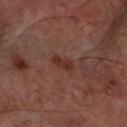The lesion was tiled from a total-body skin photograph and was not biopsied.
A roughly 15 mm field-of-view crop from a total-body skin photograph.
About 3 mm across.
A male subject, aged 63 to 67.
On the left forearm.